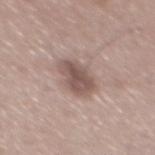{
  "biopsy_status": "not biopsied; imaged during a skin examination",
  "patient": {
    "sex": "male",
    "age_approx": 55
  },
  "lesion_size": {
    "long_diameter_mm_approx": 4.0
  },
  "lighting": "white-light",
  "image": {
    "source": "total-body photography crop",
    "field_of_view_mm": 15
  }
}